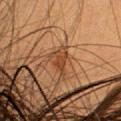Part of a total-body skin-imaging series; this lesion was reviewed on a skin check and was not flagged for biopsy. On the head or neck. An algorithmic analysis of the crop reported a lesion area of about 4 mm² and a shape-asymmetry score of about 0.4 (0 = symmetric). The software also gave border irregularity of about 4.5 on a 0–10 scale, a within-lesion color-variation index near 2/10, and radial color variation of about 0.5. The lesion's longest dimension is about 3.5 mm. A female subject in their 40s. Cropped from a total-body skin-imaging series; the visible field is about 15 mm.